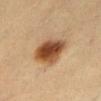| feature | finding |
|---|---|
| workup | no biopsy performed (imaged during a skin exam) |
| image-analysis metrics | roughly 15 lightness units darker than nearby skin and a normalized lesion–skin contrast near 12; a border-irregularity index near 2/10 and a within-lesion color-variation index near 6.5/10 |
| subject | female, in their mid-40s |
| body site | the abdomen |
| imaging modality | 15 mm crop, total-body photography |